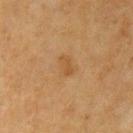No biopsy was performed on this lesion — it was imaged during a full skin examination and was not determined to be concerning. Located on the left upper arm. Measured at roughly 3 mm in maximum diameter. A female patient roughly 55 years of age. A close-up tile cropped from a whole-body skin photograph, about 15 mm across.